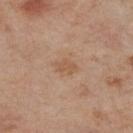Impression:
This lesion was catalogued during total-body skin photography and was not selected for biopsy.